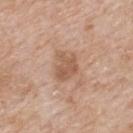Captured during whole-body skin photography for melanoma surveillance; the lesion was not biopsied. On the mid back. A male subject, aged 63 to 67. Cropped from a whole-body photographic skin survey; the tile spans about 15 mm.